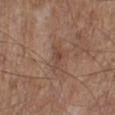– follow-up: no biopsy performed (imaged during a skin exam)
– tile lighting: white-light
– body site: the left lower leg
– patient: male, in their mid- to late 50s
– imaging modality: 15 mm crop, total-body photography
– automated lesion analysis: an area of roughly 3 mm², an outline eccentricity of about 0.9 (0 = round, 1 = elongated), and a symmetry-axis asymmetry near 0.55; border irregularity of about 5.5 on a 0–10 scale; a classifier nevus-likeness of about 0/100